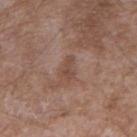| feature | finding |
|---|---|
| biopsy status | imaged on a skin check; not biopsied |
| image source | ~15 mm tile from a whole-body skin photo |
| automated metrics | an area of roughly 3 mm², an outline eccentricity of about 0.9 (0 = round, 1 = elongated), and a symmetry-axis asymmetry near 0.35; a border-irregularity index near 4/10 |
| location | the right upper arm |
| lighting | white-light |
| subject | male, aged 48 to 52 |
| lesion diameter | ~2.5 mm (longest diameter) |